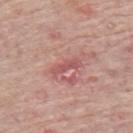biopsy_status: not biopsied; imaged during a skin examination
image:
  source: total-body photography crop
  field_of_view_mm: 15
lesion_size:
  long_diameter_mm_approx: 3.5
site: left upper arm
patient:
  sex: female
  age_approx: 70
lighting: white-light